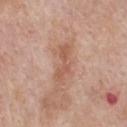Assessment:
Captured during whole-body skin photography for melanoma surveillance; the lesion was not biopsied.
Acquisition and patient details:
Located on the chest. A roughly 15 mm field-of-view crop from a total-body skin photograph. Measured at roughly 4.5 mm in maximum diameter. A male subject in their mid- to late 60s. The tile uses white-light illumination.